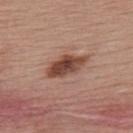Part of a total-body skin-imaging series; this lesion was reviewed on a skin check and was not flagged for biopsy.
A 15 mm close-up tile from a total-body photography series done for melanoma screening.
The lesion is located on the upper back.
The tile uses white-light illumination.
A female patient, aged around 55.